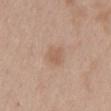Impression:
Imaged during a routine full-body skin examination; the lesion was not biopsied and no histopathology is available.
Background:
An algorithmic analysis of the crop reported a footprint of about 6 mm² and an eccentricity of roughly 0.55. The analysis additionally found a lesion color around L≈59 a*≈18 b*≈30 in CIELAB, about 7 CIELAB-L* units darker than the surrounding skin, and a normalized border contrast of about 5. It also reported a border-irregularity rating of about 1/10, internal color variation of about 2 on a 0–10 scale, and a peripheral color-asymmetry measure near 0.5. A 15 mm close-up tile from a total-body photography series done for melanoma screening. This is a white-light tile. A female subject in their 40s. The recorded lesion diameter is about 3 mm. On the mid back.